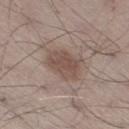Part of a total-body skin-imaging series; this lesion was reviewed on a skin check and was not flagged for biopsy. A lesion tile, about 15 mm wide, cut from a 3D total-body photograph. A male patient approximately 55 years of age. From the right thigh.